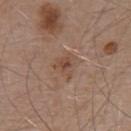Captured during whole-body skin photography for melanoma surveillance; the lesion was not biopsied. A close-up tile cropped from a whole-body skin photograph, about 15 mm across. The lesion is located on the upper back. A male patient, aged 53–57.